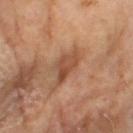follow-up: catalogued during a skin exam; not biopsied
subject: male, aged 63–67
acquisition: ~15 mm tile from a whole-body skin photo
TBP lesion metrics: a peripheral color-asymmetry measure near 1.5
lesion diameter: about 4.5 mm